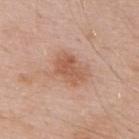Clinical impression: Captured during whole-body skin photography for melanoma surveillance; the lesion was not biopsied. Context: From the upper back. A male subject aged 53 to 57. This is a white-light tile. Longest diameter approximately 4 mm. A 15 mm close-up tile from a total-body photography series done for melanoma screening.